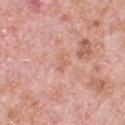Assessment:
Recorded during total-body skin imaging; not selected for excision or biopsy.
Acquisition and patient details:
The lesion is located on the chest. A lesion tile, about 15 mm wide, cut from a 3D total-body photograph. A male patient, aged around 80. Imaged with white-light lighting. The lesion-visualizer software estimated roughly 5 lightness units darker than nearby skin and a normalized lesion–skin contrast near 5. The analysis additionally found an automated nevus-likeness rating near 0 out of 100 and lesion-presence confidence of about 100/100.